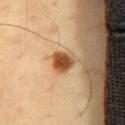Impression:
Captured during whole-body skin photography for melanoma surveillance; the lesion was not biopsied.
Background:
The lesion's longest dimension is about 3 mm. A male subject, in their 60s. A 15 mm crop from a total-body photograph taken for skin-cancer surveillance. Located on the lower back. Captured under cross-polarized illumination.